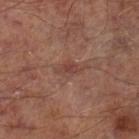  biopsy_status: not biopsied; imaged during a skin examination
  lighting: cross-polarized
  lesion_size:
    long_diameter_mm_approx: 3.0
  image:
    source: total-body photography crop
    field_of_view_mm: 15
  automated_metrics:
    cielab_L: 40
    cielab_a: 21
    cielab_b: 23
    vs_skin_darker_L: 7.0
    vs_skin_contrast_norm: 5.5
  site: left lower leg
  patient:
    sex: male
    age_approx: 65